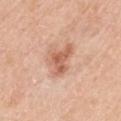On the mid back. A male subject, aged 48 to 52. Automated image analysis of the tile measured a footprint of about 7.5 mm². The analysis additionally found an automated nevus-likeness rating near 35 out of 100. This is a white-light tile. A close-up tile cropped from a whole-body skin photograph, about 15 mm across.